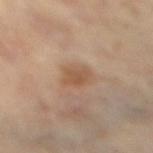Q: Automated lesion metrics?
A: a footprint of about 6.5 mm², an outline eccentricity of about 0.75 (0 = round, 1 = elongated), and a shape-asymmetry score of about 0.2 (0 = symmetric); a lesion color around L≈54 a*≈18 b*≈32 in CIELAB, about 8 CIELAB-L* units darker than the surrounding skin, and a normalized lesion–skin contrast near 6.5; border irregularity of about 2 on a 0–10 scale, internal color variation of about 2.5 on a 0–10 scale, and radial color variation of about 1
Q: What kind of image is this?
A: ~15 mm tile from a whole-body skin photo
Q: Who is the patient?
A: female, aged around 75
Q: What is the lesion's diameter?
A: about 3.5 mm
Q: Where on the body is the lesion?
A: the left lower leg